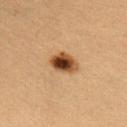The lesion was photographed on a routine skin check and not biopsied; there is no pathology result.
A 15 mm crop from a total-body photograph taken for skin-cancer surveillance.
The lesion's longest dimension is about 3.5 mm.
The lesion-visualizer software estimated a footprint of about 7 mm² and an eccentricity of roughly 0.7. The software also gave a lesion color around L≈39 a*≈20 b*≈32 in CIELAB, roughly 18 lightness units darker than nearby skin, and a lesion-to-skin contrast of about 13.5 (normalized; higher = more distinct). The software also gave a classifier nevus-likeness of about 100/100 and lesion-presence confidence of about 100/100.
On the left forearm.
This is a cross-polarized tile.
A female subject, aged 38–42.